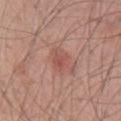Q: Is there a histopathology result?
A: total-body-photography surveillance lesion; no biopsy
Q: What did automated image analysis measure?
A: a mean CIELAB color near L≈53 a*≈25 b*≈25, about 7 CIELAB-L* units darker than the surrounding skin, and a normalized border contrast of about 5.5; a detector confidence of about 100 out of 100 that the crop contains a lesion
Q: What is the lesion's diameter?
A: ~3 mm (longest diameter)
Q: What is the anatomic site?
A: the chest
Q: Illumination type?
A: white-light
Q: Patient demographics?
A: male, in their mid-60s
Q: What kind of image is this?
A: total-body-photography crop, ~15 mm field of view